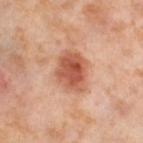| key | value |
|---|---|
| biopsy status | total-body-photography surveillance lesion; no biopsy |
| size | about 4.5 mm |
| patient | female, aged 53–57 |
| image | 15 mm crop, total-body photography |
| site | the left thigh |
| lighting | cross-polarized |